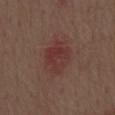Findings:
* workup: total-body-photography surveillance lesion; no biopsy
* TBP lesion metrics: an outline eccentricity of about 0.65 (0 = round, 1 = elongated) and a shape-asymmetry score of about 0.2 (0 = symmetric); a classifier nevus-likeness of about 20/100
* image source: ~15 mm crop, total-body skin-cancer survey
* patient: male, aged around 70
* body site: the mid back
* lesion size: ≈5 mm